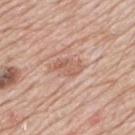Q: Was this lesion biopsied?
A: catalogued during a skin exam; not biopsied
Q: What kind of image is this?
A: ~15 mm tile from a whole-body skin photo
Q: Where on the body is the lesion?
A: the mid back
Q: Patient demographics?
A: male, approximately 80 years of age
Q: What is the lesion's diameter?
A: about 4 mm
Q: Illumination type?
A: white-light illumination